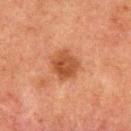notes = no biopsy performed (imaged during a skin exam)
image = total-body-photography crop, ~15 mm field of view
location = the upper back
subject = male, in their 50s
size = ≈4 mm